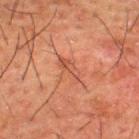automated lesion analysis: a footprint of about 5 mm² and an outline eccentricity of about 0.9 (0 = round, 1 = elongated); a normalized border contrast of about 5; a border-irregularity rating of about 3.5/10 and a peripheral color-asymmetry measure near 1.5; lesion-presence confidence of about 70/100 | lighting: cross-polarized | lesion size: ~3.5 mm (longest diameter) | patient: male, roughly 50 years of age | image: total-body-photography crop, ~15 mm field of view | location: the upper back.